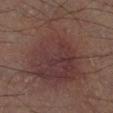Impression: No biopsy was performed on this lesion — it was imaged during a full skin examination and was not determined to be concerning. Image and clinical context: A male patient, aged approximately 50. Located on the left lower leg. A 15 mm crop from a total-body photograph taken for skin-cancer surveillance.